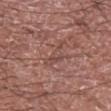The subject is a male in their mid- to late 70s. Approximately 4.5 mm at its widest. On the left upper arm. The tile uses white-light illumination. This image is a 15 mm lesion crop taken from a total-body photograph. Automated tile analysis of the lesion measured a lesion area of about 4.5 mm², an outline eccentricity of about 0.9 (0 = round, 1 = elongated), and a shape-asymmetry score of about 0.6 (0 = symmetric). It also reported a lesion color around L≈47 a*≈21 b*≈23 in CIELAB and a lesion-to-skin contrast of about 5 (normalized; higher = more distinct).